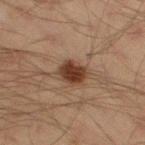Imaged during a routine full-body skin examination; the lesion was not biopsied and no histopathology is available. On the right thigh. Imaged with cross-polarized lighting. Cropped from a whole-body photographic skin survey; the tile spans about 15 mm. The subject is a male aged 43 to 47. The lesion-visualizer software estimated roughly 13 lightness units darker than nearby skin and a normalized border contrast of about 12.